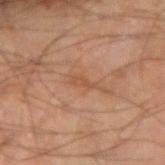workup: catalogued during a skin exam; not biopsied | site: the right forearm | patient: male, aged approximately 65 | acquisition: ~15 mm crop, total-body skin-cancer survey.